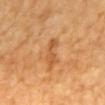{"site": "mid back", "patient": {"sex": "male", "age_approx": 65}, "lighting": "cross-polarized", "lesion_size": {"long_diameter_mm_approx": 3.5}, "image": {"source": "total-body photography crop", "field_of_view_mm": 15}}